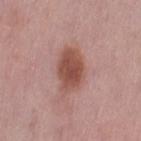{"automated_metrics": {"cielab_L": 50, "cielab_a": 23, "cielab_b": 26, "vs_skin_darker_L": 12.0, "vs_skin_contrast_norm": 9.0}, "lighting": "white-light", "site": "left thigh", "image": {"source": "total-body photography crop", "field_of_view_mm": 15}, "lesion_size": {"long_diameter_mm_approx": 5.0}, "patient": {"sex": "female", "age_approx": 50}}